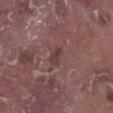Imaged during a routine full-body skin examination; the lesion was not biopsied and no histopathology is available.
The patient is a male about 75 years old.
A 15 mm crop from a total-body photograph taken for skin-cancer surveillance.
On the right lower leg.